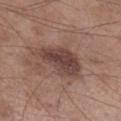No biopsy was performed on this lesion — it was imaged during a full skin examination and was not determined to be concerning. The total-body-photography lesion software estimated a lesion area of about 16 mm², a shape eccentricity near 0.8, and a shape-asymmetry score of about 0.3 (0 = symmetric). And it measured internal color variation of about 4 on a 0–10 scale. A 15 mm crop from a total-body photograph taken for skin-cancer surveillance. Approximately 6.5 mm at its widest. The patient is a male aged around 55. Imaged with white-light lighting. On the right lower leg.